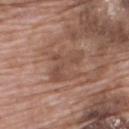Captured during whole-body skin photography for melanoma surveillance; the lesion was not biopsied. Automated tile analysis of the lesion measured a lesion area of about 8 mm², an eccentricity of roughly 0.85, and two-axis asymmetry of about 0.6. The analysis additionally found a border-irregularity rating of about 7.5/10 and a within-lesion color-variation index near 2.5/10. Cropped from a whole-body photographic skin survey; the tile spans about 15 mm. The recorded lesion diameter is about 4.5 mm. From the back. Captured under white-light illumination. The subject is a male roughly 70 years of age.